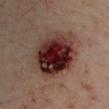– workup — no biopsy performed (imaged during a skin exam)
– subject — male, aged around 45
– lighting — cross-polarized illumination
– acquisition — ~15 mm tile from a whole-body skin photo
– image-analysis metrics — an average lesion color of about L≈29 a*≈21 b*≈20 (CIELAB); a border-irregularity index near 1.5/10 and a within-lesion color-variation index near 10/10; an automated nevus-likeness rating near 10 out of 100 and a detector confidence of about 100 out of 100 that the crop contains a lesion
– location — the left upper arm